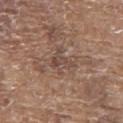{
  "biopsy_status": "not biopsied; imaged during a skin examination",
  "image": {
    "source": "total-body photography crop",
    "field_of_view_mm": 15
  },
  "site": "back",
  "lesion_size": {
    "long_diameter_mm_approx": 3.5
  },
  "patient": {
    "sex": "male",
    "age_approx": 80
  }
}